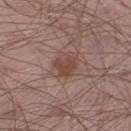Q: Was this lesion biopsied?
A: no biopsy performed (imaged during a skin exam)
Q: What is the anatomic site?
A: the leg
Q: Automated lesion metrics?
A: an area of roughly 6 mm², an eccentricity of roughly 0.5, and a symmetry-axis asymmetry near 0.2; a lesion color around L≈45 a*≈20 b*≈24 in CIELAB, about 9 CIELAB-L* units darker than the surrounding skin, and a lesion-to-skin contrast of about 7.5 (normalized; higher = more distinct); a within-lesion color-variation index near 3/10 and peripheral color asymmetry of about 1; a nevus-likeness score of about 60/100
Q: What are the patient's age and sex?
A: male, aged approximately 65
Q: Lesion size?
A: about 3 mm
Q: What is the imaging modality?
A: ~15 mm crop, total-body skin-cancer survey
Q: Illumination type?
A: white-light illumination